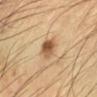{"biopsy_status": "not biopsied; imaged during a skin examination", "site": "arm", "lighting": "cross-polarized", "image": {"source": "total-body photography crop", "field_of_view_mm": 15}, "patient": {"sex": "male", "age_approx": 65}, "automated_metrics": {"area_mm2_approx": 5.5, "eccentricity": 0.7, "shape_asymmetry": 0.25, "border_irregularity_0_10": 2.5, "color_variation_0_10": 7.5, "peripheral_color_asymmetry": 3.0}, "lesion_size": {"long_diameter_mm_approx": 3.0}}